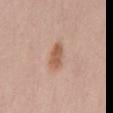This lesion was catalogued during total-body skin photography and was not selected for biopsy.
A lesion tile, about 15 mm wide, cut from a 3D total-body photograph.
The lesion is on the mid back.
A female patient, roughly 60 years of age.
Measured at roughly 3.5 mm in maximum diameter.
Automated image analysis of the tile measured a mean CIELAB color near L≈57 a*≈21 b*≈32, roughly 11 lightness units darker than nearby skin, and a lesion-to-skin contrast of about 8 (normalized; higher = more distinct).
This is a white-light tile.